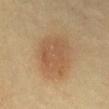No biopsy was performed on this lesion — it was imaged during a full skin examination and was not determined to be concerning. A female patient in their 60s. A close-up tile cropped from a whole-body skin photograph, about 15 mm across. Captured under cross-polarized illumination. The lesion is located on the mid back.